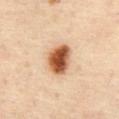workup: imaged on a skin check; not biopsied
subject: female, aged around 45
tile lighting: cross-polarized illumination
size: about 4 mm
site: the abdomen
image source: ~15 mm tile from a whole-body skin photo